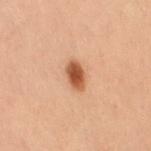biopsy_status: not biopsied; imaged during a skin examination
automated_metrics:
  border_irregularity_0_10: 2.0
  color_variation_0_10: 2.5
  peripheral_color_asymmetry: 0.5
  nevus_likeness_0_100: 100
  lesion_detection_confidence_0_100: 100
lesion_size:
  long_diameter_mm_approx: 3.0
site: right thigh
patient:
  sex: female
  age_approx: 40
image:
  source: total-body photography crop
  field_of_view_mm: 15
lighting: cross-polarized